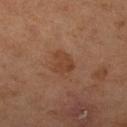Approximately 3 mm at its widest. A lesion tile, about 15 mm wide, cut from a 3D total-body photograph. Captured under cross-polarized illumination. Located on the right lower leg. A female subject, in their mid-60s. Automated tile analysis of the lesion measured a lesion–skin lightness drop of about 7. The analysis additionally found a classifier nevus-likeness of about 45/100 and a lesion-detection confidence of about 100/100.